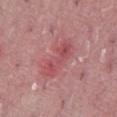Assessment:
Captured during whole-body skin photography for melanoma surveillance; the lesion was not biopsied.
Background:
On the abdomen. Longest diameter approximately 5.5 mm. A male subject aged 38–42. A close-up tile cropped from a whole-body skin photograph, about 15 mm across. An algorithmic analysis of the crop reported an outline eccentricity of about 0.95 (0 = round, 1 = elongated) and a symmetry-axis asymmetry near 0.25. And it measured border irregularity of about 4.5 on a 0–10 scale, a within-lesion color-variation index near 4.5/10, and a peripheral color-asymmetry measure near 1.5. And it measured a classifier nevus-likeness of about 0/100 and a detector confidence of about 100 out of 100 that the crop contains a lesion.